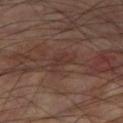Q: Was this lesion biopsied?
A: catalogued during a skin exam; not biopsied
Q: What did automated image analysis measure?
A: a footprint of about 4.5 mm² and a shape eccentricity near 0.75; internal color variation of about 1.5 on a 0–10 scale and peripheral color asymmetry of about 0.5; a classifier nevus-likeness of about 0/100
Q: What lighting was used for the tile?
A: cross-polarized illumination
Q: Lesion location?
A: the right thigh
Q: What are the patient's age and sex?
A: male, aged 58–62
Q: How large is the lesion?
A: ~3 mm (longest diameter)
Q: What is the imaging modality?
A: ~15 mm tile from a whole-body skin photo